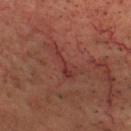{"biopsy_status": "not biopsied; imaged during a skin examination", "patient": {"sex": "male", "age_approx": 70}, "lighting": "cross-polarized", "lesion_size": {"long_diameter_mm_approx": 5.0}, "site": "head or neck", "image": {"source": "total-body photography crop", "field_of_view_mm": 15}}